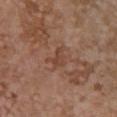Notes:
- biopsy status — imaged on a skin check; not biopsied
- subject — female, in their mid-60s
- tile lighting — white-light
- lesion size — ≈2.5 mm
- imaging modality — ~15 mm tile from a whole-body skin photo
- anatomic site — the chest